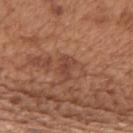Part of a total-body skin-imaging series; this lesion was reviewed on a skin check and was not flagged for biopsy. Located on the mid back. A region of skin cropped from a whole-body photographic capture, roughly 15 mm wide. About 3 mm across. The lesion-visualizer software estimated a lesion area of about 3.5 mm² and a shape-asymmetry score of about 0.5 (0 = symmetric). The software also gave a border-irregularity index near 5/10, a within-lesion color-variation index near 1.5/10, and radial color variation of about 0.5. The software also gave an automated nevus-likeness rating near 0 out of 100 and lesion-presence confidence of about 100/100. A male subject, aged 63 to 67.